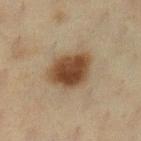Findings:
– image source: 15 mm crop, total-body photography
– lesion size: ≈5 mm
– subject: female, roughly 55 years of age
– anatomic site: the left lower leg
– tile lighting: cross-polarized illumination